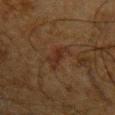Part of a total-body skin-imaging series; this lesion was reviewed on a skin check and was not flagged for biopsy. A male subject, roughly 65 years of age. Approximately 4 mm at its widest. Located on the left upper arm. Cropped from a total-body skin-imaging series; the visible field is about 15 mm. Captured under cross-polarized illumination.